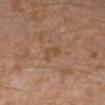workup: catalogued during a skin exam; not biopsied
imaging modality: total-body-photography crop, ~15 mm field of view
TBP lesion metrics: an area of roughly 3.5 mm², an outline eccentricity of about 0.8 (0 = round, 1 = elongated), and a shape-asymmetry score of about 0.35 (0 = symmetric); a mean CIELAB color near L≈47 a*≈19 b*≈30, roughly 5 lightness units darker than nearby skin, and a normalized border contrast of about 4.5; a border-irregularity index near 3/10 and a peripheral color-asymmetry measure near 0.5
patient: male, in their 30s
lesion size: ~3 mm (longest diameter)
site: the leg